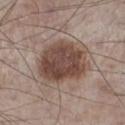notes=imaged on a skin check; not biopsied | imaging modality=15 mm crop, total-body photography | lighting=white-light illumination | patient=male, aged 58 to 62 | site=the leg | size=~6.5 mm (longest diameter).